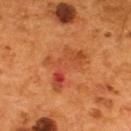biopsy_status: not biopsied; imaged during a skin examination
lighting: cross-polarized
image:
  source: total-body photography crop
  field_of_view_mm: 15
automated_metrics:
  area_mm2_approx: 13.0
  eccentricity: 0.8
  shape_asymmetry: 0.6
  cielab_L: 45
  cielab_a: 31
  cielab_b: 39
  vs_skin_darker_L: 8.0
  vs_skin_contrast_norm: 6.0
  border_irregularity_0_10: 8.0
  color_variation_0_10: 9.0
patient:
  sex: male
  age_approx: 55
site: upper back